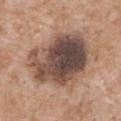Q: Lesion size?
A: about 8 mm
Q: What is the anatomic site?
A: the chest
Q: How was this image acquired?
A: 15 mm crop, total-body photography
Q: Who is the patient?
A: male, approximately 60 years of age
Q: How was the tile lit?
A: white-light illumination